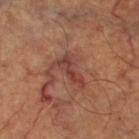Q: Lesion location?
A: the right thigh
Q: What are the patient's age and sex?
A: in their mid-60s
Q: What is the lesion's diameter?
A: ~4.5 mm (longest diameter)
Q: How was the tile lit?
A: cross-polarized
Q: What kind of image is this?
A: ~15 mm crop, total-body skin-cancer survey